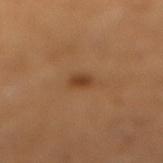Captured during whole-body skin photography for melanoma surveillance; the lesion was not biopsied. Automated tile analysis of the lesion measured a border-irregularity index near 1.5/10 and peripheral color asymmetry of about 0.5. It also reported a classifier nevus-likeness of about 90/100 and a detector confidence of about 100 out of 100 that the crop contains a lesion. The lesion is located on the leg. A female subject in their mid-50s. A 15 mm crop from a total-body photograph taken for skin-cancer surveillance.